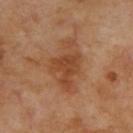Context:
Captured under cross-polarized illumination. On the upper back. A roughly 15 mm field-of-view crop from a total-body skin photograph. The subject is a male aged around 70. Automated image analysis of the tile measured an eccentricity of roughly 0.75 and a shape-asymmetry score of about 0.4 (0 = symmetric). The analysis additionally found a lesion-detection confidence of about 100/100. Approximately 6 mm at its widest.